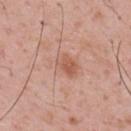biopsy status — no biopsy performed (imaged during a skin exam) | patient — male, roughly 55 years of age | anatomic site — the back | automated lesion analysis — a footprint of about 12 mm², an eccentricity of roughly 0.75, and a symmetry-axis asymmetry near 0.25; an automated nevus-likeness rating near 15 out of 100 | acquisition — total-body-photography crop, ~15 mm field of view | size — ~5 mm (longest diameter) | illumination — white-light illumination.